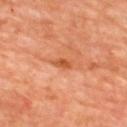Findings:
– notes · no biopsy performed (imaged during a skin exam)
– patient · male, aged 58 to 62
– anatomic site · the upper back
– image · total-body-photography crop, ~15 mm field of view
– lesion size · ~3 mm (longest diameter)
– lighting · cross-polarized